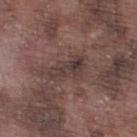| feature | finding |
|---|---|
| notes | no biopsy performed (imaged during a skin exam) |
| automated lesion analysis | a classifier nevus-likeness of about 0/100 |
| diameter | about 4.5 mm |
| location | the right lower leg |
| image | ~15 mm crop, total-body skin-cancer survey |
| subject | male, aged around 75 |